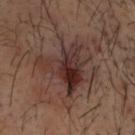Captured during whole-body skin photography for melanoma surveillance; the lesion was not biopsied.
The recorded lesion diameter is about 6 mm.
The lesion is located on the head or neck.
Captured under cross-polarized illumination.
The subject is a male aged around 40.
A lesion tile, about 15 mm wide, cut from a 3D total-body photograph.